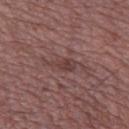This lesion was catalogued during total-body skin photography and was not selected for biopsy.
Located on the left thigh.
Longest diameter approximately 3 mm.
A male patient aged around 50.
The tile uses white-light illumination.
A roughly 15 mm field-of-view crop from a total-body skin photograph.